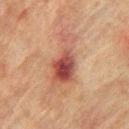This lesion was catalogued during total-body skin photography and was not selected for biopsy.